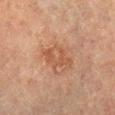Recorded during total-body skin imaging; not selected for excision or biopsy.
Automated tile analysis of the lesion measured border irregularity of about 3.5 on a 0–10 scale and a within-lesion color-variation index near 3/10. The software also gave a classifier nevus-likeness of about 15/100.
A roughly 15 mm field-of-view crop from a total-body skin photograph.
A female patient about 60 years old.
From the right lower leg.
Approximately 4 mm at its widest.